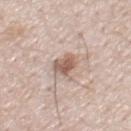Clinical impression: Imaged during a routine full-body skin examination; the lesion was not biopsied and no histopathology is available. Clinical summary: A region of skin cropped from a whole-body photographic capture, roughly 15 mm wide. From the chest. The subject is a male aged 48 to 52.